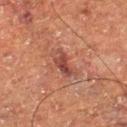No biopsy was performed on this lesion — it was imaged during a full skin examination and was not determined to be concerning. About 4 mm across. A male patient, in their mid- to late 70s. On the right thigh. A close-up tile cropped from a whole-body skin photograph, about 15 mm across.